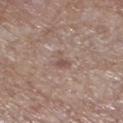Clinical impression: The lesion was tiled from a total-body skin photograph and was not biopsied. Image and clinical context: The lesion is located on the leg. The total-body-photography lesion software estimated a lesion area of about 4 mm² and an eccentricity of roughly 0.6. The analysis additionally found an average lesion color of about L≈53 a*≈16 b*≈22 (CIELAB), a lesion–skin lightness drop of about 7, and a normalized lesion–skin contrast near 5. The software also gave border irregularity of about 5 on a 0–10 scale, internal color variation of about 1 on a 0–10 scale, and a peripheral color-asymmetry measure near 0.5. The analysis additionally found a classifier nevus-likeness of about 0/100 and lesion-presence confidence of about 100/100. A region of skin cropped from a whole-body photographic capture, roughly 15 mm wide. The lesion's longest dimension is about 3 mm. A male subject, aged approximately 85 or older.